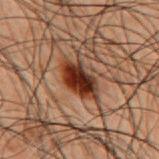<record>
<lesion_size>
  <long_diameter_mm_approx>5.0</long_diameter_mm_approx>
</lesion_size>
<site>mid back</site>
<lighting>cross-polarized</lighting>
<patient>
  <sex>male</sex>
  <age_approx>50</age_approx>
</patient>
<image>
  <source>total-body photography crop</source>
  <field_of_view_mm>15</field_of_view_mm>
</image>
</record>